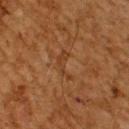Longest diameter approximately 3.5 mm. Automated image analysis of the tile measured a footprint of about 3.5 mm² and a shape eccentricity near 0.9. It also reported an average lesion color of about L≈32 a*≈20 b*≈30 (CIELAB), a lesion–skin lightness drop of about 5, and a lesion-to-skin contrast of about 5 (normalized; higher = more distinct). It also reported a border-irregularity index near 9/10, a color-variation rating of about 0/10, and a peripheral color-asymmetry measure near 0. The software also gave a nevus-likeness score of about 0/100 and lesion-presence confidence of about 90/100. On the back. This is a cross-polarized tile. The patient is a male about 60 years old. A 15 mm crop from a total-body photograph taken for skin-cancer surveillance.